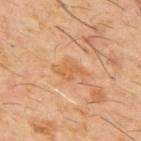No biopsy was performed on this lesion — it was imaged during a full skin examination and was not determined to be concerning. The patient is a male in their 60s. Captured under cross-polarized illumination. The lesion-visualizer software estimated a lesion area of about 6 mm², a shape eccentricity near 0.85, and a symmetry-axis asymmetry near 0.25. It also reported border irregularity of about 3 on a 0–10 scale. Measured at roughly 4 mm in maximum diameter. Cropped from a total-body skin-imaging series; the visible field is about 15 mm. On the back.